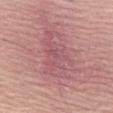No biopsy was performed on this lesion — it was imaged during a full skin examination and was not determined to be concerning.
On the abdomen.
The patient is a male aged around 80.
A 15 mm close-up tile from a total-body photography series done for melanoma screening.
Imaged with white-light lighting.